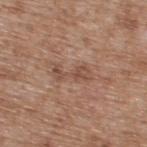notes: total-body-photography surveillance lesion; no biopsy
image-analysis metrics: a footprint of about 5.5 mm², an outline eccentricity of about 0.95 (0 = round, 1 = elongated), and two-axis asymmetry of about 0.45; a color-variation rating of about 2/10 and a peripheral color-asymmetry measure near 0.5; a classifier nevus-likeness of about 0/100
site: the back
patient: male, in their mid- to late 60s
imaging modality: 15 mm crop, total-body photography
diameter: about 4.5 mm